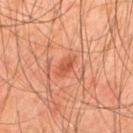The recorded lesion diameter is about 2 mm. A male patient, roughly 45 years of age. Automated tile analysis of the lesion measured an eccentricity of roughly 0.85 and two-axis asymmetry of about 0.35. The analysis additionally found border irregularity of about 3.5 on a 0–10 scale and a color-variation rating of about 0.5/10. On the mid back. This is a cross-polarized tile. Cropped from a total-body skin-imaging series; the visible field is about 15 mm.